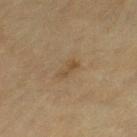tile lighting: cross-polarized; image source: total-body-photography crop, ~15 mm field of view; location: the left thigh; lesion size: ≈2.5 mm; subject: female, about 55 years old.